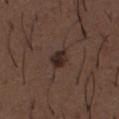The lesion was photographed on a routine skin check and not biopsied; there is no pathology result. The lesion is on the front of the torso. About 3 mm across. A male patient aged around 50. This is a white-light tile. Automated image analysis of the tile measured a border-irregularity rating of about 2.5/10, a color-variation rating of about 4/10, and a peripheral color-asymmetry measure near 1.5. It also reported a lesion-detection confidence of about 100/100. A roughly 15 mm field-of-view crop from a total-body skin photograph.